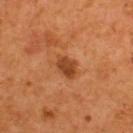This lesion was catalogued during total-body skin photography and was not selected for biopsy. Imaged with cross-polarized lighting. A roughly 15 mm field-of-view crop from a total-body skin photograph. On the back. A male subject, aged 53 to 57. The total-body-photography lesion software estimated an area of roughly 5.5 mm², an outline eccentricity of about 0.75 (0 = round, 1 = elongated), and two-axis asymmetry of about 0.2. The analysis additionally found a normalized border contrast of about 8. The lesion's longest dimension is about 3 mm.